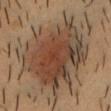| feature | finding |
|---|---|
| notes | total-body-photography surveillance lesion; no biopsy |
| illumination | cross-polarized illumination |
| acquisition | 15 mm crop, total-body photography |
| lesion size | ≈10.5 mm |
| patient | male, about 40 years old |
| location | the head or neck |